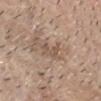No biopsy was performed on this lesion — it was imaged during a full skin examination and was not determined to be concerning.
The lesion is located on the head or neck.
The recorded lesion diameter is about 3.5 mm.
A close-up tile cropped from a whole-body skin photograph, about 15 mm across.
The tile uses white-light illumination.
The total-body-photography lesion software estimated a footprint of about 4.5 mm² and an eccentricity of roughly 0.8. And it measured an average lesion color of about L≈53 a*≈16 b*≈27 (CIELAB), a lesion–skin lightness drop of about 8, and a normalized lesion–skin contrast near 5.5. The software also gave a border-irregularity rating of about 5.5/10, a color-variation rating of about 1/10, and radial color variation of about 0.5. It also reported a classifier nevus-likeness of about 0/100.
A male patient aged 78 to 82.